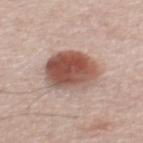The lesion was photographed on a routine skin check and not biopsied; there is no pathology result. The lesion's longest dimension is about 5.5 mm. The tile uses white-light illumination. Automated image analysis of the tile measured a lesion color around L≈52 a*≈21 b*≈25 in CIELAB, about 17 CIELAB-L* units darker than the surrounding skin, and a lesion-to-skin contrast of about 11 (normalized; higher = more distinct). And it measured a border-irregularity index near 1.5/10, a color-variation rating of about 8/10, and a peripheral color-asymmetry measure near 2.5. The subject is a male aged 53 to 57. A 15 mm close-up tile from a total-body photography series done for melanoma screening. On the mid back.